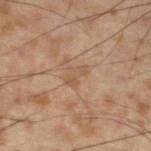Q: Is there a histopathology result?
A: imaged on a skin check; not biopsied
Q: Automated lesion metrics?
A: an area of roughly 3.5 mm² and a symmetry-axis asymmetry near 0.45; a lesion color around L≈55 a*≈18 b*≈33 in CIELAB, roughly 6 lightness units darker than nearby skin, and a normalized lesion–skin contrast near 5
Q: What is the lesion's diameter?
A: about 3 mm
Q: Lesion location?
A: the leg
Q: What lighting was used for the tile?
A: cross-polarized illumination
Q: What are the patient's age and sex?
A: male, aged around 55
Q: What is the imaging modality?
A: total-body-photography crop, ~15 mm field of view